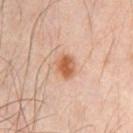Clinical impression: No biopsy was performed on this lesion — it was imaged during a full skin examination and was not determined to be concerning. Clinical summary: A 15 mm crop from a total-body photograph taken for skin-cancer surveillance. This is a cross-polarized tile. A male patient approximately 35 years of age. The lesion is located on the chest.